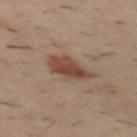Imaged during a routine full-body skin examination; the lesion was not biopsied and no histopathology is available. This is a cross-polarized tile. A region of skin cropped from a whole-body photographic capture, roughly 15 mm wide. The lesion is located on the upper back. The lesion-visualizer software estimated an outline eccentricity of about 0.8 (0 = round, 1 = elongated) and a symmetry-axis asymmetry near 0.25. The software also gave a mean CIELAB color near L≈43 a*≈19 b*≈26, about 11 CIELAB-L* units darker than the surrounding skin, and a normalized lesion–skin contrast near 8.5. It also reported a border-irregularity index near 3/10, a within-lesion color-variation index near 3.5/10, and peripheral color asymmetry of about 1. A male subject aged around 40. The lesion's longest dimension is about 4.5 mm.